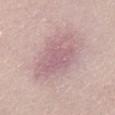<record>
  <biopsy_status>not biopsied; imaged during a skin examination</biopsy_status>
  <lighting>white-light</lighting>
  <site>chest</site>
  <image>
    <source>total-body photography crop</source>
    <field_of_view_mm>15</field_of_view_mm>
  </image>
  <automated_metrics>
    <eccentricity>0.7</eccentricity>
    <shape_asymmetry>0.35</shape_asymmetry>
    <cielab_L>60</cielab_L>
    <cielab_a>21</cielab_a>
    <cielab_b>16</cielab_b>
    <vs_skin_darker_L>8.0</vs_skin_darker_L>
    <vs_skin_contrast_norm>5.5</vs_skin_contrast_norm>
    <border_irregularity_0_10>4.0</border_irregularity_0_10>
    <color_variation_0_10>2.5</color_variation_0_10>
    <peripheral_color_asymmetry>1.0</peripheral_color_asymmetry>
  </automated_metrics>
  <patient>
    <sex>female</sex>
    <age_approx>70</age_approx>
  </patient>
  <lesion_size>
    <long_diameter_mm_approx>4.5</long_diameter_mm_approx>
  </lesion_size>
</record>